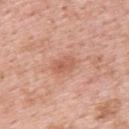Q: Was a biopsy performed?
A: total-body-photography surveillance lesion; no biopsy
Q: What did automated image analysis measure?
A: a mean CIELAB color near L≈59 a*≈26 b*≈31, a lesion–skin lightness drop of about 8, and a normalized border contrast of about 6; a border-irregularity index near 2/10, a within-lesion color-variation index near 3.5/10, and peripheral color asymmetry of about 1.5
Q: Illumination type?
A: white-light
Q: Patient demographics?
A: female, approximately 50 years of age
Q: How was this image acquired?
A: ~15 mm tile from a whole-body skin photo
Q: How large is the lesion?
A: about 3 mm
Q: What is the anatomic site?
A: the upper back